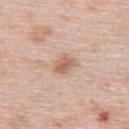notes: no biopsy performed (imaged during a skin exam) | patient: female, about 65 years old | diameter: ≈3 mm | illumination: white-light | acquisition: total-body-photography crop, ~15 mm field of view | image-analysis metrics: a lesion area of about 5 mm², a shape eccentricity near 0.7, and two-axis asymmetry of about 0.3; a lesion color around L≈62 a*≈19 b*≈30 in CIELAB and a lesion–skin lightness drop of about 10; a classifier nevus-likeness of about 70/100 and a lesion-detection confidence of about 100/100 | anatomic site: the upper back.